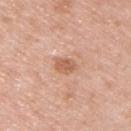A region of skin cropped from a whole-body photographic capture, roughly 15 mm wide.
This is a white-light tile.
Located on the upper back.
Measured at roughly 3 mm in maximum diameter.
A male subject, in their mid-40s.